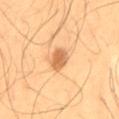* notes: no biopsy performed (imaged during a skin exam)
* lesion diameter: ≈3.5 mm
* lighting: cross-polarized illumination
* site: the mid back
* imaging modality: 15 mm crop, total-body photography
* subject: male, approximately 55 years of age